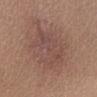  biopsy_status: not biopsied; imaged during a skin examination
  lesion_size:
    long_diameter_mm_approx: 6.5
  site: right lower leg
  patient:
    sex: female
    age_approx: 30
  image:
    source: total-body photography crop
    field_of_view_mm: 15
  lighting: white-light